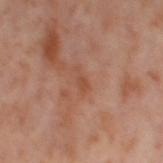workup: total-body-photography surveillance lesion; no biopsy
subject: female, aged around 55
lesion diameter: ~2.5 mm (longest diameter)
anatomic site: the leg
imaging modality: ~15 mm crop, total-body skin-cancer survey
lighting: cross-polarized illumination